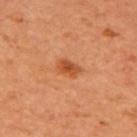The lesion was tiled from a total-body skin photograph and was not biopsied. The tile uses cross-polarized illumination. The subject is female. The lesion is on the upper back. A region of skin cropped from a whole-body photographic capture, roughly 15 mm wide. Automated image analysis of the tile measured an area of roughly 4.5 mm², a shape eccentricity near 0.8, and a shape-asymmetry score of about 0.2 (0 = symmetric). And it measured a within-lesion color-variation index near 3.5/10 and a peripheral color-asymmetry measure near 1.